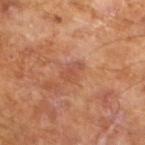The lesion's longest dimension is about 2.5 mm. Automated tile analysis of the lesion measured an average lesion color of about L≈50 a*≈25 b*≈32 (CIELAB), a lesion–skin lightness drop of about 7, and a lesion-to-skin contrast of about 5 (normalized; higher = more distinct). The software also gave a nevus-likeness score of about 0/100 and a lesion-detection confidence of about 100/100. This is a cross-polarized tile. A male subject about 65 years old. A 15 mm crop from a total-body photograph taken for skin-cancer surveillance.